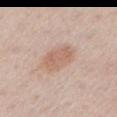• workup — total-body-photography surveillance lesion; no biopsy
• image source — ~15 mm crop, total-body skin-cancer survey
• subject — male, aged 58–62
• lighting — white-light
• lesion size — ≈4.5 mm
• anatomic site — the left upper arm